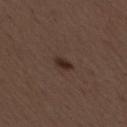Clinical impression:
The lesion was photographed on a routine skin check and not biopsied; there is no pathology result.
Clinical summary:
The total-body-photography lesion software estimated a mean CIELAB color near L≈27 a*≈16 b*≈21, roughly 9 lightness units darker than nearby skin, and a normalized lesion–skin contrast near 9.5. And it measured a nevus-likeness score of about 95/100 and a lesion-detection confidence of about 100/100. The lesion's longest dimension is about 2.5 mm. The tile uses white-light illumination. On the mid back. A region of skin cropped from a whole-body photographic capture, roughly 15 mm wide. A female subject aged 48–52.